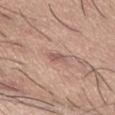Findings:
- workup: total-body-photography surveillance lesion; no biopsy
- imaging modality: total-body-photography crop, ~15 mm field of view
- patient: male, aged 63–67
- lighting: white-light
- lesion diameter: ~2.5 mm (longest diameter)
- site: the abdomen